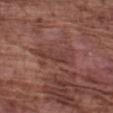Impression:
The lesion was photographed on a routine skin check and not biopsied; there is no pathology result.
Clinical summary:
A close-up tile cropped from a whole-body skin photograph, about 15 mm across. Imaged with white-light lighting. Automated image analysis of the tile measured an average lesion color of about L≈40 a*≈22 b*≈23 (CIELAB) and a lesion–skin lightness drop of about 6. A male patient aged 73–77. The lesion is on the left forearm. Approximately 5 mm at its widest.